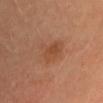This lesion was catalogued during total-body skin photography and was not selected for biopsy. Captured under cross-polarized illumination. A roughly 15 mm field-of-view crop from a total-body skin photograph. The lesion-visualizer software estimated an eccentricity of roughly 0.7 and a symmetry-axis asymmetry near 0.4. The software also gave a border-irregularity rating of about 4/10 and internal color variation of about 2.5 on a 0–10 scale. The analysis additionally found a nevus-likeness score of about 15/100 and a detector confidence of about 100 out of 100 that the crop contains a lesion. Located on the head or neck. Measured at roughly 4 mm in maximum diameter. The patient is a female aged approximately 35.